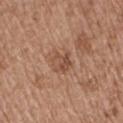| key | value |
|---|---|
| illumination | white-light illumination |
| anatomic site | the leg |
| TBP lesion metrics | a mean CIELAB color near L≈49 a*≈21 b*≈30 and a normalized lesion–skin contrast near 6 |
| size | about 3 mm |
| imaging modality | ~15 mm tile from a whole-body skin photo |
| patient | female, aged around 75 |